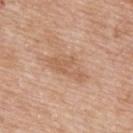<case>
  <biopsy_status>not biopsied; imaged during a skin examination</biopsy_status>
</case>